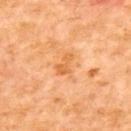| field | value |
|---|---|
| biopsy status | catalogued during a skin exam; not biopsied |
| tile lighting | cross-polarized illumination |
| image source | ~15 mm crop, total-body skin-cancer survey |
| patient | male, about 55 years old |
| location | the upper back |